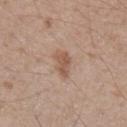biopsy status: no biopsy performed (imaged during a skin exam); lighting: white-light; subject: male, aged around 45; size: about 3.5 mm; image: 15 mm crop, total-body photography; automated metrics: a lesion area of about 4.5 mm², an outline eccentricity of about 0.8 (0 = round, 1 = elongated), and a symmetry-axis asymmetry near 0.4; location: the arm.